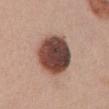| key | value |
|---|---|
| follow-up | total-body-photography surveillance lesion; no biopsy |
| anatomic site | the chest |
| image | ~15 mm tile from a whole-body skin photo |
| subject | female, aged 33 to 37 |